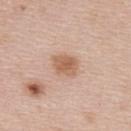notes: imaged on a skin check; not biopsied
lesion diameter: about 3 mm
imaging modality: ~15 mm crop, total-body skin-cancer survey
anatomic site: the upper back
patient: female, approximately 50 years of age
automated metrics: a footprint of about 6.5 mm² and a shape-asymmetry score of about 0.2 (0 = symmetric); lesion-presence confidence of about 100/100